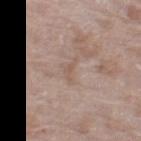{"biopsy_status": "not biopsied; imaged during a skin examination", "patient": {"sex": "female", "age_approx": 75}, "lesion_size": {"long_diameter_mm_approx": 2.5}, "image": {"source": "total-body photography crop", "field_of_view_mm": 15}, "site": "left thigh", "lighting": "white-light"}